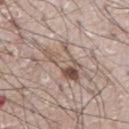The lesion was tiled from a total-body skin photograph and was not biopsied. From the abdomen. Longest diameter approximately 8 mm. The subject is a male roughly 75 years of age. A region of skin cropped from a whole-body photographic capture, roughly 15 mm wide. Imaged with white-light lighting. The lesion-visualizer software estimated an eccentricity of roughly 0.95. It also reported a mean CIELAB color near L≈55 a*≈14 b*≈23 and a lesion-to-skin contrast of about 7 (normalized; higher = more distinct). It also reported a nevus-likeness score of about 5/100 and a detector confidence of about 75 out of 100 that the crop contains a lesion.